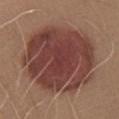<record>
<lesion_size>
  <long_diameter_mm_approx>9.5</long_diameter_mm_approx>
</lesion_size>
<lighting>white-light</lighting>
<site>leg</site>
<patient>
  <sex>male</sex>
  <age_approx>30</age_approx>
</patient>
<image>
  <source>total-body photography crop</source>
  <field_of_view_mm>15</field_of_view_mm>
</image>
</record>